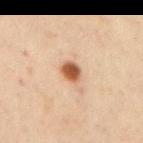Impression: Imaged during a routine full-body skin examination; the lesion was not biopsied and no histopathology is available. Image and clinical context: A male patient, approximately 50 years of age. The tile uses cross-polarized illumination. Automated tile analysis of the lesion measured a detector confidence of about 100 out of 100 that the crop contains a lesion. This image is a 15 mm lesion crop taken from a total-body photograph. On the right upper arm. Longest diameter approximately 2.5 mm.